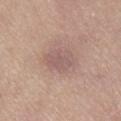notes=imaged on a skin check; not biopsied
acquisition=~15 mm tile from a whole-body skin photo
location=the right lower leg
patient=female, about 65 years old
diameter=about 4 mm
tile lighting=white-light illumination